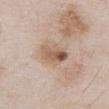follow-up: imaged on a skin check; not biopsied